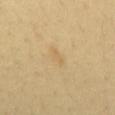Captured during whole-body skin photography for melanoma surveillance; the lesion was not biopsied. This is a cross-polarized tile. Automated image analysis of the tile measured a lesion area of about 2.5 mm², a shape eccentricity near 0.8, and two-axis asymmetry of about 0.35. And it measured a border-irregularity index near 3.5/10, a within-lesion color-variation index near 0.5/10, and a peripheral color-asymmetry measure near 0. A lesion tile, about 15 mm wide, cut from a 3D total-body photograph. A male subject, aged around 45. Approximately 2 mm at its widest. The lesion is located on the mid back.